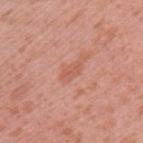<lesion>
<biopsy_status>not biopsied; imaged during a skin examination</biopsy_status>
<lesion_size>
  <long_diameter_mm_approx>2.5</long_diameter_mm_approx>
</lesion_size>
<site>upper back</site>
<image>
  <source>total-body photography crop</source>
  <field_of_view_mm>15</field_of_view_mm>
</image>
<automated_metrics>
  <area_mm2_approx>3.0</area_mm2_approx>
  <eccentricity>0.85</eccentricity>
  <shape_asymmetry>0.3</shape_asymmetry>
  <border_irregularity_0_10>3.0</border_irregularity_0_10>
  <color_variation_0_10>1.5</color_variation_0_10>
  <peripheral_color_asymmetry>0.5</peripheral_color_asymmetry>
  <lesion_detection_confidence_0_100>100</lesion_detection_confidence_0_100>
</automated_metrics>
<patient>
  <sex>female</sex>
  <age_approx>40</age_approx>
</patient>
<lighting>white-light</lighting>
</lesion>